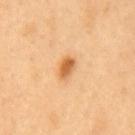A male subject, aged around 50. The lesion is located on the mid back. A lesion tile, about 15 mm wide, cut from a 3D total-body photograph.